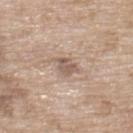Case summary:
- biopsy status · no biopsy performed (imaged during a skin exam)
- body site · the upper back
- subject · female, approximately 75 years of age
- automated metrics · a border-irregularity rating of about 3.5/10, a within-lesion color-variation index near 3/10, and radial color variation of about 1
- imaging modality · 15 mm crop, total-body photography